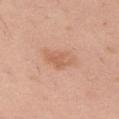Impression:
Imaged during a routine full-body skin examination; the lesion was not biopsied and no histopathology is available.
Background:
A female subject, in their 30s. Approximately 4.5 mm at its widest. A 15 mm crop from a total-body photograph taken for skin-cancer surveillance. From the left thigh.